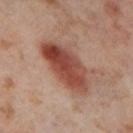The lesion was photographed on a routine skin check and not biopsied; there is no pathology result. This is a cross-polarized tile. The lesion is on the leg. A 15 mm close-up extracted from a 3D total-body photography capture. A female patient, about 55 years old. Automated tile analysis of the lesion measured a border-irregularity index near 2.5/10 and a within-lesion color-variation index near 8/10. The lesion's longest dimension is about 7.5 mm.